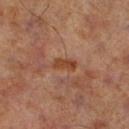Q: Was this lesion biopsied?
A: total-body-photography surveillance lesion; no biopsy
Q: What kind of image is this?
A: ~15 mm crop, total-body skin-cancer survey
Q: Who is the patient?
A: male, aged 68 to 72
Q: Lesion location?
A: the left lower leg